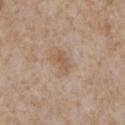The lesion was photographed on a routine skin check and not biopsied; there is no pathology result. From the chest. A male patient, aged approximately 65. A 15 mm close-up tile from a total-body photography series done for melanoma screening. Approximately 3 mm at its widest. This is a white-light tile.